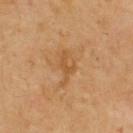Assessment: Part of a total-body skin-imaging series; this lesion was reviewed on a skin check and was not flagged for biopsy. Image and clinical context: A male patient, aged around 70. The lesion-visualizer software estimated an area of roughly 4.5 mm² and a symmetry-axis asymmetry near 0.5. The software also gave a nevus-likeness score of about 0/100 and a detector confidence of about 100 out of 100 that the crop contains a lesion. A region of skin cropped from a whole-body photographic capture, roughly 15 mm wide. This is a cross-polarized tile. The lesion is located on the upper back. Longest diameter approximately 4 mm.